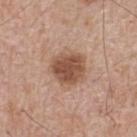Assessment: Part of a total-body skin-imaging series; this lesion was reviewed on a skin check and was not flagged for biopsy. Background: The lesion is on the upper back. Measured at roughly 4 mm in maximum diameter. The total-body-photography lesion software estimated a lesion color around L≈51 a*≈20 b*≈30 in CIELAB and roughly 13 lightness units darker than nearby skin. It also reported a border-irregularity rating of about 1.5/10 and peripheral color asymmetry of about 1. The analysis additionally found a nevus-likeness score of about 70/100 and lesion-presence confidence of about 100/100. This is a white-light tile. A male subject, about 65 years old. A roughly 15 mm field-of-view crop from a total-body skin photograph.